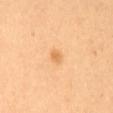{"biopsy_status": "not biopsied; imaged during a skin examination", "site": "mid back", "image": {"source": "total-body photography crop", "field_of_view_mm": 15}, "patient": {"sex": "female", "age_approx": 55}, "automated_metrics": {"cielab_L": 70, "cielab_a": 24, "cielab_b": 47, "vs_skin_darker_L": 9.0, "vs_skin_contrast_norm": 6.5, "border_irregularity_0_10": 2.0, "color_variation_0_10": 0.5, "peripheral_color_asymmetry": 0.0}}